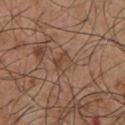Part of a total-body skin-imaging series; this lesion was reviewed on a skin check and was not flagged for biopsy.
Located on the chest.
Longest diameter approximately 3 mm.
The total-body-photography lesion software estimated a lesion area of about 4.5 mm², a shape eccentricity near 0.65, and a symmetry-axis asymmetry near 0.35. The analysis additionally found roughly 6 lightness units darker than nearby skin and a normalized lesion–skin contrast near 5.5.
A region of skin cropped from a whole-body photographic capture, roughly 15 mm wide.
The patient is a male in their mid- to late 50s.